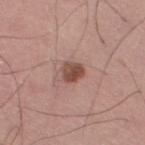follow-up — catalogued during a skin exam; not biopsied
tile lighting — white-light illumination
subject — male, aged around 70
anatomic site — the right thigh
image — total-body-photography crop, ~15 mm field of view